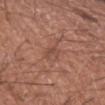Background: A 15 mm close-up tile from a total-body photography series done for melanoma screening. A male patient, about 60 years old. This is a white-light tile. The lesion is on the arm.